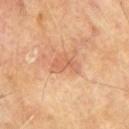Clinical impression:
This lesion was catalogued during total-body skin photography and was not selected for biopsy.
Context:
The lesion is located on the right upper arm. The subject is a male aged around 65. Automated tile analysis of the lesion measured a footprint of about 5.5 mm² and an outline eccentricity of about 0.8 (0 = round, 1 = elongated). The analysis additionally found a border-irregularity index near 5/10 and a within-lesion color-variation index near 3/10. And it measured a detector confidence of about 100 out of 100 that the crop contains a lesion. A 15 mm close-up tile from a total-body photography series done for melanoma screening. Measured at roughly 3.5 mm in maximum diameter. This is a cross-polarized tile.